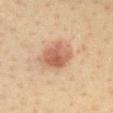  biopsy_status: not biopsied; imaged during a skin examination
  lighting: cross-polarized
  patient:
    sex: female
    age_approx: 40
  site: chest
  lesion_size:
    long_diameter_mm_approx: 4.5
  image:
    source: total-body photography crop
    field_of_view_mm: 15